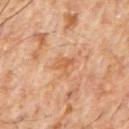No biopsy was performed on this lesion — it was imaged during a full skin examination and was not determined to be concerning.
A lesion tile, about 15 mm wide, cut from a 3D total-body photograph.
The tile uses cross-polarized illumination.
A male subject aged 58 to 62.
Approximately 2.5 mm at its widest.
An algorithmic analysis of the crop reported a shape eccentricity near 0.75 and a shape-asymmetry score of about 0.3 (0 = symmetric). It also reported about 7 CIELAB-L* units darker than the surrounding skin and a normalized border contrast of about 5.5. It also reported a nevus-likeness score of about 0/100 and a detector confidence of about 100 out of 100 that the crop contains a lesion.
From the chest.